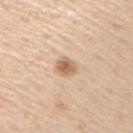follow-up: no biopsy performed (imaged during a skin exam)
subject: male, about 60 years old
anatomic site: the arm
size: ≈2.5 mm
illumination: white-light illumination
image source: 15 mm crop, total-body photography
image-analysis metrics: a symmetry-axis asymmetry near 0.25; border irregularity of about 2.5 on a 0–10 scale, a color-variation rating of about 3.5/10, and a peripheral color-asymmetry measure near 1; an automated nevus-likeness rating near 90 out of 100